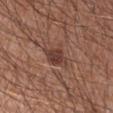The lesion was tiled from a total-body skin photograph and was not biopsied. This is a white-light tile. The lesion-visualizer software estimated a border-irregularity index near 3.5/10 and a peripheral color-asymmetry measure near 1. It also reported a nevus-likeness score of about 70/100 and lesion-presence confidence of about 100/100. Located on the arm. A roughly 15 mm field-of-view crop from a total-body skin photograph. A male subject, aged 28 to 32. The recorded lesion diameter is about 3.5 mm.